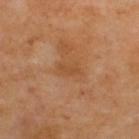Clinical impression: Captured during whole-body skin photography for melanoma surveillance; the lesion was not biopsied. Acquisition and patient details: A roughly 15 mm field-of-view crop from a total-body skin photograph. From the upper back. A male patient about 70 years old.